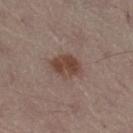Background: The tile uses white-light illumination. The lesion's longest dimension is about 4 mm. A male patient aged around 55. The total-body-photography lesion software estimated a lesion area of about 8 mm², a shape eccentricity near 0.75, and a symmetry-axis asymmetry near 0.15. The analysis additionally found a lesion color around L≈43 a*≈18 b*≈24 in CIELAB, about 10 CIELAB-L* units darker than the surrounding skin, and a normalized lesion–skin contrast near 8.5. And it measured a nevus-likeness score of about 95/100 and a detector confidence of about 100 out of 100 that the crop contains a lesion. The lesion is on the right thigh. A lesion tile, about 15 mm wide, cut from a 3D total-body photograph.